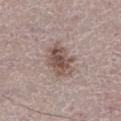notes=catalogued during a skin exam; not biopsied | body site=the leg | subject=male, aged 68 to 72 | lighting=white-light illumination | imaging modality=15 mm crop, total-body photography | automated metrics=a footprint of about 10 mm², a shape eccentricity near 0.8, and a symmetry-axis asymmetry near 0.25; a lesion–skin lightness drop of about 12 and a normalized lesion–skin contrast near 8.5.